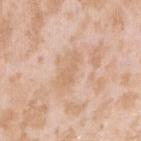Q: What is the imaging modality?
A: ~15 mm crop, total-body skin-cancer survey
Q: Illumination type?
A: white-light
Q: How large is the lesion?
A: ≈3.5 mm
Q: Who is the patient?
A: female, approximately 25 years of age
Q: Lesion location?
A: the right upper arm